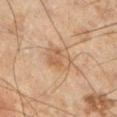biopsy status: total-body-photography surveillance lesion; no biopsy